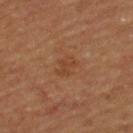This lesion was catalogued during total-body skin photography and was not selected for biopsy. The lesion's longest dimension is about 2.5 mm. From the upper back. Imaged with cross-polarized lighting. A roughly 15 mm field-of-view crop from a total-body skin photograph. A male patient, aged 53–57. Automated image analysis of the tile measured a shape eccentricity near 0.75 and two-axis asymmetry of about 0.3. The analysis additionally found a mean CIELAB color near L≈37 a*≈20 b*≈29 and roughly 5 lightness units darker than nearby skin. And it measured a color-variation rating of about 1/10 and peripheral color asymmetry of about 0.5.